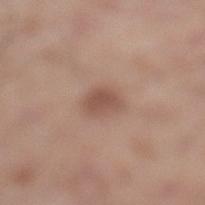This lesion was catalogued during total-body skin photography and was not selected for biopsy.
About 3 mm across.
On the leg.
A male subject, aged 53 to 57.
A lesion tile, about 15 mm wide, cut from a 3D total-body photograph.
The tile uses white-light illumination.